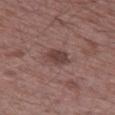Impression: Captured during whole-body skin photography for melanoma surveillance; the lesion was not biopsied. Acquisition and patient details: The lesion-visualizer software estimated an eccentricity of roughly 0.65. The software also gave a detector confidence of about 100 out of 100 that the crop contains a lesion. Located on the leg. Longest diameter approximately 3 mm. A male subject in their 50s. This is a white-light tile. A roughly 15 mm field-of-view crop from a total-body skin photograph.